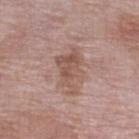Clinical impression:
The lesion was photographed on a routine skin check and not biopsied; there is no pathology result.
Background:
Automated tile analysis of the lesion measured border irregularity of about 6.5 on a 0–10 scale, internal color variation of about 3.5 on a 0–10 scale, and peripheral color asymmetry of about 1. The analysis additionally found an automated nevus-likeness rating near 0 out of 100 and a lesion-detection confidence of about 100/100. The recorded lesion diameter is about 5.5 mm. A female patient approximately 70 years of age. The lesion is on the left lower leg. A 15 mm crop from a total-body photograph taken for skin-cancer surveillance.